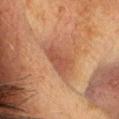workup — no biopsy performed (imaged during a skin exam)
site — the head or neck
image-analysis metrics — an average lesion color of about L≈41 a*≈21 b*≈27 (CIELAB), roughly 8 lightness units darker than nearby skin, and a normalized lesion–skin contrast near 6.5; a nevus-likeness score of about 30/100 and lesion-presence confidence of about 100/100
subject — male, aged 48 to 52
image source — total-body-photography crop, ~15 mm field of view